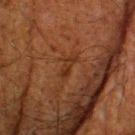Captured during whole-body skin photography for melanoma surveillance; the lesion was not biopsied.
From the head or neck.
This is a cross-polarized tile.
Approximately 2.5 mm at its widest.
A male subject, in their 60s.
Cropped from a whole-body photographic skin survey; the tile spans about 15 mm.